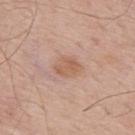This lesion was catalogued during total-body skin photography and was not selected for biopsy. Imaged with white-light lighting. A roughly 15 mm field-of-view crop from a total-body skin photograph. A male patient aged 63–67. Located on the upper back. Approximately 3 mm at its widest.